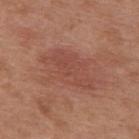Q: Is there a histopathology result?
A: catalogued during a skin exam; not biopsied
Q: Patient demographics?
A: female, about 40 years old
Q: What is the imaging modality?
A: total-body-photography crop, ~15 mm field of view
Q: What did automated image analysis measure?
A: a footprint of about 16 mm² and a shape-asymmetry score of about 0.35 (0 = symmetric); an average lesion color of about L≈48 a*≈25 b*≈28 (CIELAB), roughly 6 lightness units darker than nearby skin, and a normalized lesion–skin contrast near 4.5; a nevus-likeness score of about 0/100
Q: Lesion size?
A: about 6.5 mm
Q: What is the anatomic site?
A: the back